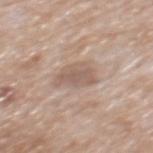Clinical impression:
No biopsy was performed on this lesion — it was imaged during a full skin examination and was not determined to be concerning.
Acquisition and patient details:
A close-up tile cropped from a whole-body skin photograph, about 15 mm across. A male subject aged 63–67. On the mid back.